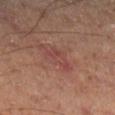Q: Lesion size?
A: ≈3.5 mm
Q: What is the imaging modality?
A: ~15 mm tile from a whole-body skin photo
Q: What are the patient's age and sex?
A: male, approximately 50 years of age
Q: What is the anatomic site?
A: the right lower leg